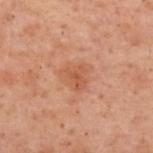  biopsy_status: not biopsied; imaged during a skin examination
  image:
    source: total-body photography crop
    field_of_view_mm: 15
  lighting: cross-polarized
  automated_metrics:
    eccentricity: 0.4
    shape_asymmetry: 0.35
    cielab_L: 46
    cielab_a: 22
    cielab_b: 30
    vs_skin_darker_L: 6.0
    vs_skin_contrast_norm: 5.5
  patient:
    sex: female
    age_approx: 55
  lesion_size:
    long_diameter_mm_approx: 3.5
  site: back